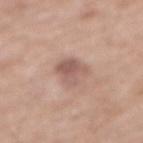A male patient, aged approximately 70. The lesion is located on the abdomen. A close-up tile cropped from a whole-body skin photograph, about 15 mm across. This is a white-light tile. Measured at roughly 4 mm in maximum diameter. Automated tile analysis of the lesion measured an average lesion color of about L≈56 a*≈19 b*≈24 (CIELAB). And it measured a border-irregularity rating of about 3.5/10 and internal color variation of about 4.5 on a 0–10 scale. It also reported a classifier nevus-likeness of about 10/100.